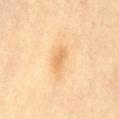workup: no biopsy performed (imaged during a skin exam)
subject: female, in their 80s
body site: the lower back
imaging modality: 15 mm crop, total-body photography